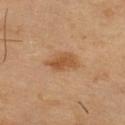No biopsy was performed on this lesion — it was imaged during a full skin examination and was not determined to be concerning.